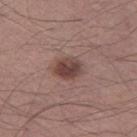Assessment: Imaged during a routine full-body skin examination; the lesion was not biopsied and no histopathology is available. Background: On the left thigh. The lesion-visualizer software estimated a footprint of about 7 mm², a shape eccentricity near 0.65, and two-axis asymmetry of about 0.1. And it measured a lesion color around L≈43 a*≈19 b*≈21 in CIELAB, a lesion–skin lightness drop of about 12, and a normalized lesion–skin contrast near 9. The software also gave a within-lesion color-variation index near 4/10 and a peripheral color-asymmetry measure near 1.5. And it measured a classifier nevus-likeness of about 90/100 and lesion-presence confidence of about 100/100. Cropped from a total-body skin-imaging series; the visible field is about 15 mm. A male subject, aged 53–57. Measured at roughly 3.5 mm in maximum diameter.